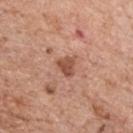Clinical impression: No biopsy was performed on this lesion — it was imaged during a full skin examination and was not determined to be concerning. Background: The tile uses white-light illumination. A region of skin cropped from a whole-body photographic capture, roughly 15 mm wide. From the front of the torso. A male patient, aged approximately 70. The recorded lesion diameter is about 2.5 mm.